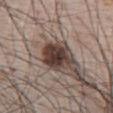No biopsy was performed on this lesion — it was imaged during a full skin examination and was not determined to be concerning. The lesion's longest dimension is about 4 mm. This is a white-light tile. The lesion is located on the abdomen. A 15 mm close-up extracted from a 3D total-body photography capture. A male patient, roughly 65 years of age.